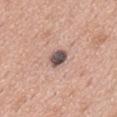This lesion was catalogued during total-body skin photography and was not selected for biopsy.
This image is a 15 mm lesion crop taken from a total-body photograph.
The tile uses white-light illumination.
From the chest.
About 3 mm across.
A male subject, aged 53–57.